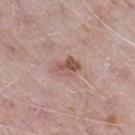The lesion was tiled from a total-body skin photograph and was not biopsied. About 3 mm across. Automated image analysis of the tile measured a lesion area of about 5 mm², a shape eccentricity near 0.7, and two-axis asymmetry of about 0.3. It also reported a lesion color around L≈53 a*≈21 b*≈24 in CIELAB and a normalized lesion–skin contrast near 7.5. The analysis additionally found an automated nevus-likeness rating near 50 out of 100 and a lesion-detection confidence of about 100/100. A male subject, aged 48 to 52. This is a white-light tile. A 15 mm close-up tile from a total-body photography series done for melanoma screening. Located on the right thigh.